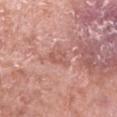Findings:
- follow-up · catalogued during a skin exam; not biopsied
- size · ≈2.5 mm
- body site · the leg
- tile lighting · white-light
- patient · male, aged approximately 55
- imaging modality · ~15 mm crop, total-body skin-cancer survey